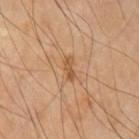This lesion was catalogued during total-body skin photography and was not selected for biopsy. The lesion's longest dimension is about 3 mm. The lesion is located on the left upper arm. Imaged with cross-polarized lighting. The subject is a male aged approximately 65. A region of skin cropped from a whole-body photographic capture, roughly 15 mm wide.